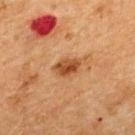The lesion was tiled from a total-body skin photograph and was not biopsied.